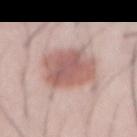biopsy status: catalogued during a skin exam; not biopsied
imaging modality: ~15 mm crop, total-body skin-cancer survey
location: the abdomen
patient: male, aged 23–27
lighting: white-light
automated lesion analysis: an area of roughly 21 mm² and an outline eccentricity of about 0.55 (0 = round, 1 = elongated); a border-irregularity index near 2.5/10, internal color variation of about 4 on a 0–10 scale, and radial color variation of about 1; a nevus-likeness score of about 95/100